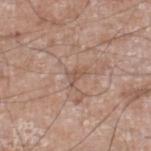Clinical impression:
This lesion was catalogued during total-body skin photography and was not selected for biopsy.
Image and clinical context:
The subject is a male aged 58–62. The lesion is located on the right lower leg. A lesion tile, about 15 mm wide, cut from a 3D total-body photograph. Imaged with white-light lighting. The lesion's longest dimension is about 2.5 mm.